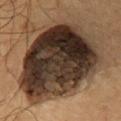Assessment: Imaged during a routine full-body skin examination; the lesion was not biopsied and no histopathology is available.